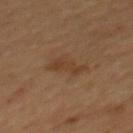Imaged during a routine full-body skin examination; the lesion was not biopsied and no histopathology is available. The total-body-photography lesion software estimated a classifier nevus-likeness of about 10/100 and a lesion-detection confidence of about 100/100. A lesion tile, about 15 mm wide, cut from a 3D total-body photograph. The subject is a male aged approximately 55. Measured at roughly 4 mm in maximum diameter. Located on the mid back.